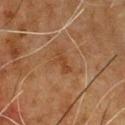Captured during whole-body skin photography for melanoma surveillance; the lesion was not biopsied.
From the chest.
A lesion tile, about 15 mm wide, cut from a 3D total-body photograph.
The recorded lesion diameter is about 3 mm.
The patient is a male aged 58–62.